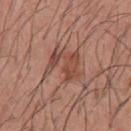workup: no biopsy performed (imaged during a skin exam)
subject: male, aged 43 to 47
illumination: white-light illumination
location: the upper back
automated lesion analysis: a footprint of about 11 mm², an outline eccentricity of about 0.6 (0 = round, 1 = elongated), and a shape-asymmetry score of about 0.5 (0 = symmetric); a mean CIELAB color near L≈47 a*≈22 b*≈28, a lesion–skin lightness drop of about 9, and a normalized border contrast of about 7; a border-irregularity rating of about 7.5/10, a within-lesion color-variation index near 3.5/10, and radial color variation of about 1
image source: ~15 mm tile from a whole-body skin photo
lesion size: ≈4 mm